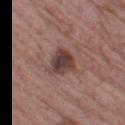biopsy_status: not biopsied; imaged during a skin examination
lesion_size:
  long_diameter_mm_approx: 4.0
automated_metrics:
  cielab_L: 41
  cielab_a: 18
  cielab_b: 21
  vs_skin_darker_L: 12.0
  vs_skin_contrast_norm: 9.5
image:
  source: total-body photography crop
  field_of_view_mm: 15
site: leg
patient:
  sex: male
  age_approx: 65